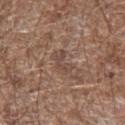  biopsy_status: not biopsied; imaged during a skin examination
  patient:
    sex: male
    age_approx: 70
  image:
    source: total-body photography crop
    field_of_view_mm: 15
  lighting: white-light
  site: left forearm
  automated_metrics:
    eccentricity: 0.8
    shape_asymmetry: 0.55
    cielab_L: 45
    cielab_a: 17
    cielab_b: 24
    vs_skin_darker_L: 7.0
    vs_skin_contrast_norm: 5.5
    border_irregularity_0_10: 6.5
    color_variation_0_10: 0.0
    peripheral_color_asymmetry: 0.0
    nevus_likeness_0_100: 0
    lesion_detection_confidence_0_100: 60